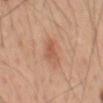Imaged during a routine full-body skin examination; the lesion was not biopsied and no histopathology is available.
A male subject, in their mid- to late 60s.
Located on the front of the torso.
A 15 mm crop from a total-body photograph taken for skin-cancer surveillance.